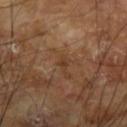Clinical impression:
No biopsy was performed on this lesion — it was imaged during a full skin examination and was not determined to be concerning.
Image and clinical context:
Imaged with cross-polarized lighting. The lesion is located on the left forearm. Automated tile analysis of the lesion measured a footprint of about 1 mm², a shape eccentricity near 0.6, and a symmetry-axis asymmetry near 0.3. It also reported border irregularity of about 2.5 on a 0–10 scale and internal color variation of about 0 on a 0–10 scale. It also reported a nevus-likeness score of about 0/100. Cropped from a whole-body photographic skin survey; the tile spans about 15 mm. A male subject, in their mid-60s. Approximately 1 mm at its widest.